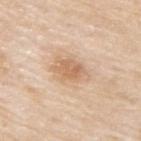Impression:
Imaged during a routine full-body skin examination; the lesion was not biopsied and no histopathology is available.
Context:
A male subject, roughly 80 years of age. Captured under white-light illumination. A 15 mm crop from a total-body photograph taken for skin-cancer surveillance. Longest diameter approximately 4.5 mm. Automated image analysis of the tile measured a lesion area of about 9.5 mm² and a shape eccentricity near 0.8. The lesion is located on the upper back.